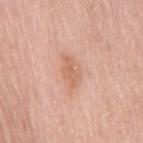* workup — no biopsy performed (imaged during a skin exam)
* lesion diameter — ~3.5 mm (longest diameter)
* image-analysis metrics — a footprint of about 5.5 mm², an eccentricity of roughly 0.85, and a shape-asymmetry score of about 0.3 (0 = symmetric); border irregularity of about 3.5 on a 0–10 scale and internal color variation of about 2 on a 0–10 scale
* subject — female, approximately 60 years of age
* lighting — white-light illumination
* acquisition — ~15 mm crop, total-body skin-cancer survey
* location — the mid back